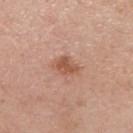workup — no biopsy performed (imaged during a skin exam) | site — the chest | tile lighting — white-light | image — ~15 mm crop, total-body skin-cancer survey | patient — male, aged approximately 55 | lesion diameter — ≈3 mm.